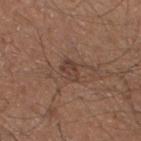Q: Is there a histopathology result?
A: total-body-photography surveillance lesion; no biopsy
Q: Patient demographics?
A: male, aged around 50
Q: What is the anatomic site?
A: the left lower leg
Q: What is the imaging modality?
A: 15 mm crop, total-body photography
Q: What lighting was used for the tile?
A: white-light illumination
Q: Automated lesion metrics?
A: an area of roughly 4 mm² and an outline eccentricity of about 0.75 (0 = round, 1 = elongated); peripheral color asymmetry of about 1